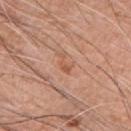Assessment:
No biopsy was performed on this lesion — it was imaged during a full skin examination and was not determined to be concerning.
Image and clinical context:
A close-up tile cropped from a whole-body skin photograph, about 15 mm across. The lesion is located on the chest. A male patient roughly 60 years of age.